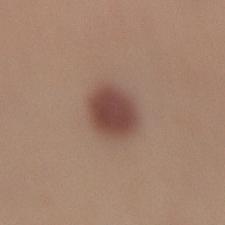* follow-up: no biopsy performed (imaged during a skin exam)
* size: ~3.5 mm (longest diameter)
* lighting: white-light
* body site: the left lower leg
* automated lesion analysis: a lesion color around L≈45 a*≈20 b*≈24 in CIELAB and about 13 CIELAB-L* units darker than the surrounding skin
* subject: male, about 30 years old
* image source: 15 mm crop, total-body photography